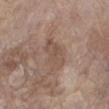Q: Where on the body is the lesion?
A: the right lower leg
Q: Patient demographics?
A: female, aged approximately 85 or older
Q: Illumination type?
A: white-light illumination
Q: What kind of image is this?
A: 15 mm crop, total-body photography
Q: How large is the lesion?
A: ≈4.5 mm